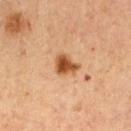biopsy status: imaged on a skin check; not biopsied | lesion diameter: ≈3 mm | patient: male, aged 63–67 | tile lighting: cross-polarized | imaging modality: ~15 mm tile from a whole-body skin photo | site: the mid back.